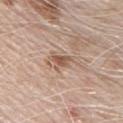Clinical impression:
Part of a total-body skin-imaging series; this lesion was reviewed on a skin check and was not flagged for biopsy.
Image and clinical context:
The lesion is on the front of the torso. A roughly 15 mm field-of-view crop from a total-body skin photograph. A male subject about 70 years old. Longest diameter approximately 2.5 mm. Captured under white-light illumination. An algorithmic analysis of the crop reported a border-irregularity rating of about 5/10. It also reported an automated nevus-likeness rating near 0 out of 100.